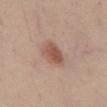Context:
Imaged with white-light lighting. An algorithmic analysis of the crop reported a lesion area of about 7.5 mm², an outline eccentricity of about 0.7 (0 = round, 1 = elongated), and a shape-asymmetry score of about 0.2 (0 = symmetric). And it measured a mean CIELAB color near L≈54 a*≈21 b*≈27 and a normalized border contrast of about 7.5. It also reported a within-lesion color-variation index near 4/10 and radial color variation of about 1.5. The software also gave a nevus-likeness score of about 95/100 and a lesion-detection confidence of about 100/100. Longest diameter approximately 3.5 mm. A close-up tile cropped from a whole-body skin photograph, about 15 mm across. The lesion is on the abdomen. A male subject aged around 40.